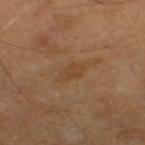- notes: catalogued during a skin exam; not biopsied
- lesion diameter: about 3 mm
- illumination: cross-polarized illumination
- patient: male, roughly 65 years of age
- acquisition: 15 mm crop, total-body photography